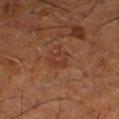No biopsy was performed on this lesion — it was imaged during a full skin examination and was not determined to be concerning. A 15 mm close-up extracted from a 3D total-body photography capture. A male subject, in their mid- to late 60s. The lesion is on the left lower leg. The tile uses cross-polarized illumination. The total-body-photography lesion software estimated a footprint of about 4.5 mm², an outline eccentricity of about 0.65 (0 = round, 1 = elongated), and two-axis asymmetry of about 0.25. The analysis additionally found a classifier nevus-likeness of about 5/100. The lesion's longest dimension is about 2.5 mm.